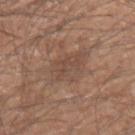<record>
<lighting>white-light</lighting>
<lesion_size>
  <long_diameter_mm_approx>5.5</long_diameter_mm_approx>
</lesion_size>
<site>left forearm</site>
<patient>
  <sex>male</sex>
  <age_approx>50</age_approx>
</patient>
<image>
  <source>total-body photography crop</source>
  <field_of_view_mm>15</field_of_view_mm>
</image>
</record>